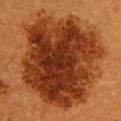{
  "biopsy_status": "not biopsied; imaged during a skin examination",
  "patient": {
    "sex": "female",
    "age_approx": 55
  },
  "lesion_size": {
    "long_diameter_mm_approx": 11.5
  },
  "lighting": "cross-polarized",
  "site": "upper back",
  "automated_metrics": {
    "area_mm2_approx": 90.0,
    "eccentricity": 0.35,
    "shape_asymmetry": 0.15,
    "cielab_L": 31,
    "cielab_a": 25,
    "cielab_b": 34,
    "vs_skin_darker_L": 14.0,
    "border_irregularity_0_10": 2.5,
    "color_variation_0_10": 7.0,
    "peripheral_color_asymmetry": 2.0
  },
  "image": {
    "source": "total-body photography crop",
    "field_of_view_mm": 15
  }
}